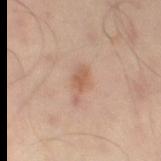{
  "biopsy_status": "not biopsied; imaged during a skin examination",
  "site": "left thigh",
  "image": {
    "source": "total-body photography crop",
    "field_of_view_mm": 15
  },
  "patient": {
    "sex": "male",
    "age_approx": 50
  },
  "lighting": "cross-polarized",
  "lesion_size": {
    "long_diameter_mm_approx": 3.5
  }
}